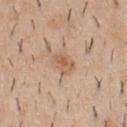Notes:
- biopsy status: imaged on a skin check; not biopsied
- TBP lesion metrics: an area of roughly 5 mm², an eccentricity of roughly 0.7, and two-axis asymmetry of about 0.25; a lesion color around L≈59 a*≈20 b*≈32 in CIELAB and a lesion–skin lightness drop of about 9; a border-irregularity rating of about 2/10, a color-variation rating of about 3/10, and peripheral color asymmetry of about 1
- patient: male, about 40 years old
- location: the chest
- acquisition: 15 mm crop, total-body photography